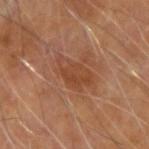workup — no biopsy performed (imaged during a skin exam)
body site — the arm
lesion diameter — ~4.5 mm (longest diameter)
imaging modality — ~15 mm crop, total-body skin-cancer survey
patient — male, in their 70s